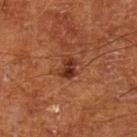Assessment:
Imaged during a routine full-body skin examination; the lesion was not biopsied and no histopathology is available.
Context:
Automated tile analysis of the lesion measured a mean CIELAB color near L≈33 a*≈25 b*≈30, a lesion–skin lightness drop of about 11, and a normalized border contrast of about 10. The analysis additionally found a border-irregularity index near 3.5/10, a color-variation rating of about 4/10, and radial color variation of about 1.5. The analysis additionally found a nevus-likeness score of about 80/100 and lesion-presence confidence of about 100/100. A male patient, aged approximately 65. The tile uses cross-polarized illumination. A close-up tile cropped from a whole-body skin photograph, about 15 mm across. On the left lower leg.